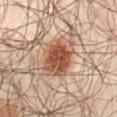Clinical summary:
Approximately 5 mm at its widest. The tile uses cross-polarized illumination. A male subject approximately 65 years of age. This image is a 15 mm lesion crop taken from a total-body photograph. Located on the front of the torso.